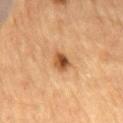Clinical impression:
No biopsy was performed on this lesion — it was imaged during a full skin examination and was not determined to be concerning.
Context:
A male patient, aged 83 or older. A 15 mm close-up tile from a total-body photography series done for melanoma screening. The tile uses cross-polarized illumination. Approximately 2.5 mm at its widest. On the mid back. The total-body-photography lesion software estimated a footprint of about 4.5 mm², a shape eccentricity near 0.6, and two-axis asymmetry of about 0.2. And it measured a lesion–skin lightness drop of about 13 and a normalized border contrast of about 9.5. It also reported a within-lesion color-variation index near 6/10 and radial color variation of about 2.